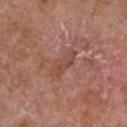| field | value |
|---|---|
| follow-up | imaged on a skin check; not biopsied |
| location | the chest |
| tile lighting | white-light |
| patient | male, approximately 65 years of age |
| acquisition | ~15 mm tile from a whole-body skin photo |